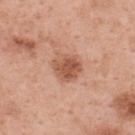| feature | finding |
|---|---|
| imaging modality | 15 mm crop, total-body photography |
| body site | the back |
| subject | female, aged 48–52 |